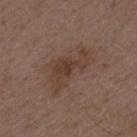  biopsy_status: not biopsied; imaged during a skin examination
  image:
    source: total-body photography crop
    field_of_view_mm: 15
  automated_metrics:
    area_mm2_approx: 16.0
    shape_asymmetry: 0.35
    cielab_L: 40
    cielab_a: 17
    cielab_b: 24
    vs_skin_darker_L: 6.0
    vs_skin_contrast_norm: 6.0
  lighting: white-light
  lesion_size:
    long_diameter_mm_approx: 6.5
  patient:
    sex: male
    age_approx: 50
  site: mid back